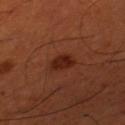Findings:
- notes — catalogued during a skin exam; not biopsied
- lighting — cross-polarized illumination
- automated metrics — a lesion-detection confidence of about 100/100
- subject — male, in their 50s
- lesion size — ~3 mm (longest diameter)
- imaging modality — ~15 mm tile from a whole-body skin photo
- body site — the left thigh